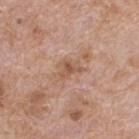Assessment:
The lesion was tiled from a total-body skin photograph and was not biopsied.
Context:
A male patient, in their mid-60s. This is a white-light tile. A 15 mm crop from a total-body photograph taken for skin-cancer surveillance. The lesion's longest dimension is about 3 mm. The lesion is located on the upper back.